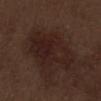Clinical impression: This lesion was catalogued during total-body skin photography and was not selected for biopsy. Background: Longest diameter approximately 8 mm. A 15 mm close-up tile from a total-body photography series done for melanoma screening. Automated image analysis of the tile measured a footprint of about 26 mm² and an eccentricity of roughly 0.8. It also reported a border-irregularity index near 3.5/10, a within-lesion color-variation index near 4/10, and peripheral color asymmetry of about 1.5. It also reported an automated nevus-likeness rating near 0 out of 100. The lesion is on the abdomen. A male patient, aged 68–72.